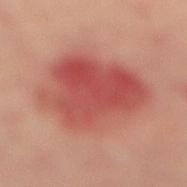Clinical summary:
The lesion-visualizer software estimated an area of roughly 47 mm². And it measured a border-irregularity rating of about 2/10, internal color variation of about 7 on a 0–10 scale, and peripheral color asymmetry of about 2.5. The recorded lesion diameter is about 8 mm. A lesion tile, about 15 mm wide, cut from a 3D total-body photograph. From the right lower leg. A female subject, roughly 65 years of age. The tile uses cross-polarized illumination.
Diagnosis:
Histopathological examination showed a superficial basal cell carcinoma, classified as a malignant skin lesion.